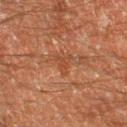  biopsy_status: not biopsied; imaged during a skin examination
  lighting: cross-polarized
  lesion_size:
    long_diameter_mm_approx: 2.5
  image:
    source: total-body photography crop
    field_of_view_mm: 15
  site: leg
  patient:
    sex: male
    age_approx: 60
  automated_metrics:
    eccentricity: 0.75
    shape_asymmetry: 0.65
    nevus_likeness_0_100: 0
    lesion_detection_confidence_0_100: 100